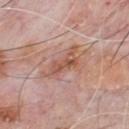follow-up — catalogued during a skin exam; not biopsied
lesion diameter — ~5.5 mm (longest diameter)
patient — male, approximately 60 years of age
image source — ~15 mm tile from a whole-body skin photo
automated lesion analysis — a border-irregularity index near 5.5/10, internal color variation of about 5 on a 0–10 scale, and radial color variation of about 2; a classifier nevus-likeness of about 0/100 and a detector confidence of about 100 out of 100 that the crop contains a lesion
illumination — white-light illumination
site — the front of the torso